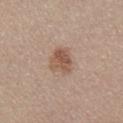A 15 mm close-up tile from a total-body photography series done for melanoma screening. A male patient, aged approximately 55. The lesion is on the chest. Captured under white-light illumination.